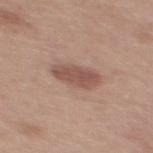Q: Is there a histopathology result?
A: total-body-photography surveillance lesion; no biopsy
Q: What kind of image is this?
A: ~15 mm crop, total-body skin-cancer survey
Q: What did automated image analysis measure?
A: an automated nevus-likeness rating near 65 out of 100 and a lesion-detection confidence of about 100/100
Q: What is the anatomic site?
A: the upper back
Q: How was the tile lit?
A: white-light illumination
Q: Patient demographics?
A: male, about 30 years old